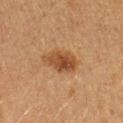* follow-up: imaged on a skin check; not biopsied
* patient: female, aged 38–42
* image: 15 mm crop, total-body photography
* body site: the left forearm